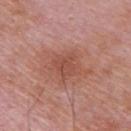notes: catalogued during a skin exam; not biopsied | tile lighting: white-light | imaging modality: total-body-photography crop, ~15 mm field of view | subject: male, in their mid-60s | anatomic site: the back | TBP lesion metrics: a footprint of about 5 mm² and a symmetry-axis asymmetry near 0.35; an average lesion color of about L≈49 a*≈26 b*≈28 (CIELAB), a lesion–skin lightness drop of about 7, and a lesion-to-skin contrast of about 5.5 (normalized; higher = more distinct) | lesion diameter: ~2.5 mm (longest diameter).